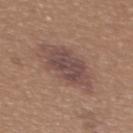No biopsy was performed on this lesion — it was imaged during a full skin examination and was not determined to be concerning. Captured under white-light illumination. A female patient aged approximately 40. A 15 mm crop from a total-body photograph taken for skin-cancer surveillance. The lesion is on the upper back. About 6 mm across.